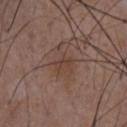The lesion was photographed on a routine skin check and not biopsied; there is no pathology result. Measured at roughly 3 mm in maximum diameter. The lesion is on the chest. A roughly 15 mm field-of-view crop from a total-body skin photograph. A male patient aged 48–52.